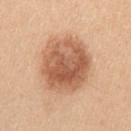<case>
  <biopsy_status>not biopsied; imaged during a skin examination</biopsy_status>
  <automated_metrics>
    <area_mm2_approx>32.0</area_mm2_approx>
    <eccentricity>0.4</eccentricity>
    <shape_asymmetry>0.1</shape_asymmetry>
    <cielab_L>59</cielab_L>
    <cielab_a>23</cielab_a>
    <cielab_b>34</cielab_b>
    <vs_skin_darker_L>14.0</vs_skin_darker_L>
    <vs_skin_contrast_norm>9.0</vs_skin_contrast_norm>
  </automated_metrics>
  <lighting>white-light</lighting>
  <patient>
    <sex>female</sex>
    <age_approx>40</age_approx>
  </patient>
  <lesion_size>
    <long_diameter_mm_approx>6.5</long_diameter_mm_approx>
  </lesion_size>
  <image>
    <source>total-body photography crop</source>
    <field_of_view_mm>15</field_of_view_mm>
  </image>
  <site>right upper arm</site>
</case>